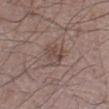{"biopsy_status": "not biopsied; imaged during a skin examination", "image": {"source": "total-body photography crop", "field_of_view_mm": 15}, "automated_metrics": {"area_mm2_approx": 6.0, "eccentricity": 0.75, "shape_asymmetry": 0.15, "nevus_likeness_0_100": 5}, "lesion_size": {"long_diameter_mm_approx": 3.5}, "patient": {"sex": "male", "age_approx": 50}, "site": "left thigh"}